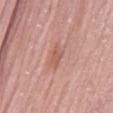Recorded during total-body skin imaging; not selected for excision or biopsy. A 15 mm close-up tile from a total-body photography series done for melanoma screening. About 3 mm across. This is a white-light tile. From the mid back. A male subject aged 53–57.